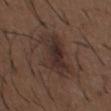Captured during whole-body skin photography for melanoma surveillance; the lesion was not biopsied.